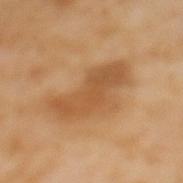No biopsy was performed on this lesion — it was imaged during a full skin examination and was not determined to be concerning. The lesion is on the mid back. Approximately 7 mm at its widest. Automated tile analysis of the lesion measured an area of roughly 15 mm², an outline eccentricity of about 0.9 (0 = round, 1 = elongated), and a symmetry-axis asymmetry near 0.4. The software also gave an average lesion color of about L≈54 a*≈22 b*≈40 (CIELAB), a lesion–skin lightness drop of about 9, and a lesion-to-skin contrast of about 6.5 (normalized; higher = more distinct). The analysis additionally found lesion-presence confidence of about 100/100. A roughly 15 mm field-of-view crop from a total-body skin photograph. The subject is a female aged 53–57. Imaged with cross-polarized lighting.